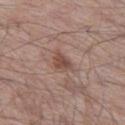Impression:
Recorded during total-body skin imaging; not selected for excision or biopsy.
Clinical summary:
On the left thigh. Captured under white-light illumination. A male patient, aged 63–67. The lesion-visualizer software estimated a footprint of about 4.5 mm², an outline eccentricity of about 0.75 (0 = round, 1 = elongated), and a shape-asymmetry score of about 0.3 (0 = symmetric). And it measured about 10 CIELAB-L* units darker than the surrounding skin. This image is a 15 mm lesion crop taken from a total-body photograph.